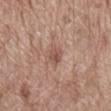Notes:
– follow-up — no biopsy performed (imaged during a skin exam)
– body site — the back
– lighting — white-light illumination
– acquisition — total-body-photography crop, ~15 mm field of view
– patient — male, aged 68–72